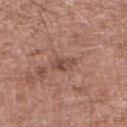The lesion was photographed on a routine skin check and not biopsied; there is no pathology result.
The lesion's longest dimension is about 2.5 mm.
A 15 mm close-up tile from a total-body photography series done for melanoma screening.
Captured under white-light illumination.
On the left lower leg.
A male subject, roughly 60 years of age.